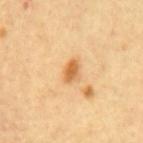• workup — imaged on a skin check; not biopsied
• patient — about 60 years old
• image source — ~15 mm crop, total-body skin-cancer survey
• site — the mid back